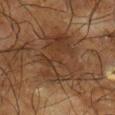Case summary:
- biopsy status — catalogued during a skin exam; not biopsied
- lesion diameter — about 8 mm
- acquisition — 15 mm crop, total-body photography
- subject — male, roughly 65 years of age
- location — the right lower leg